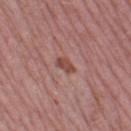This lesion was catalogued during total-body skin photography and was not selected for biopsy. A 15 mm close-up tile from a total-body photography series done for melanoma screening. From the left thigh. The lesion-visualizer software estimated a border-irregularity rating of about 2.5/10, internal color variation of about 2.5 on a 0–10 scale, and radial color variation of about 1. The analysis additionally found a classifier nevus-likeness of about 20/100. This is a white-light tile. The patient is a female aged approximately 65. The lesion's longest dimension is about 2.5 mm.